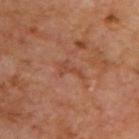Recorded during total-body skin imaging; not selected for excision or biopsy.
Longest diameter approximately 3 mm.
Automated image analysis of the tile measured a mean CIELAB color near L≈44 a*≈25 b*≈31, a lesion–skin lightness drop of about 6, and a lesion-to-skin contrast of about 5 (normalized; higher = more distinct). The software also gave a border-irregularity rating of about 8/10, internal color variation of about 0 on a 0–10 scale, and peripheral color asymmetry of about 0. And it measured a nevus-likeness score of about 0/100 and lesion-presence confidence of about 95/100.
The lesion is located on the upper back.
A lesion tile, about 15 mm wide, cut from a 3D total-body photograph.
The patient is a male about 70 years old.